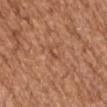Recorded during total-body skin imaging; not selected for excision or biopsy. This is a white-light tile. Cropped from a total-body skin-imaging series; the visible field is about 15 mm. A female patient aged approximately 75. About 3 mm across. The lesion is located on the left upper arm.